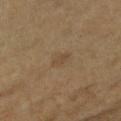Impression:
Imaged during a routine full-body skin examination; the lesion was not biopsied and no histopathology is available.
Clinical summary:
Measured at roughly 2.5 mm in maximum diameter. A 15 mm close-up extracted from a 3D total-body photography capture. From the right forearm. A female patient approximately 60 years of age.